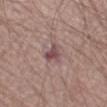Imaged during a routine full-body skin examination; the lesion was not biopsied and no histopathology is available. Captured under white-light illumination. A male subject aged 63 to 67. The recorded lesion diameter is about 3 mm. Cropped from a total-body skin-imaging series; the visible field is about 15 mm. From the left thigh. Automated image analysis of the tile measured border irregularity of about 4 on a 0–10 scale and a peripheral color-asymmetry measure near 2.